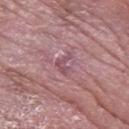The lesion was tiled from a total-body skin photograph and was not biopsied. An algorithmic analysis of the crop reported an area of roughly 3.5 mm² and a symmetry-axis asymmetry near 0.6. Imaged with white-light lighting. A male subject aged 58 to 62. Located on the left forearm. A region of skin cropped from a whole-body photographic capture, roughly 15 mm wide.